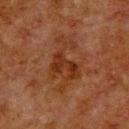patient:
  sex: male
  age_approx: 80
site: upper back
lesion_size:
  long_diameter_mm_approx: 7.0
automated_metrics:
  eccentricity: 0.85
  shape_asymmetry: 0.55
lighting: cross-polarized
image:
  source: total-body photography crop
  field_of_view_mm: 15